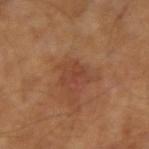<tbp_lesion>
<biopsy_status>not biopsied; imaged during a skin examination</biopsy_status>
<lighting>cross-polarized</lighting>
<site>left arm</site>
<image>
  <source>total-body photography crop</source>
  <field_of_view_mm>15</field_of_view_mm>
</image>
<patient>
  <sex>male</sex>
  <age_approx>65</age_approx>
</patient>
</tbp_lesion>